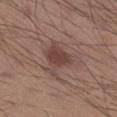Notes:
– workup · catalogued during a skin exam; not biopsied
– TBP lesion metrics · a border-irregularity rating of about 6.5/10
– body site · the right lower leg
– patient · male, aged around 30
– image source · ~15 mm tile from a whole-body skin photo
– illumination · white-light